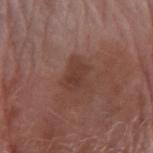Recorded during total-body skin imaging; not selected for excision or biopsy. From the arm. Captured under white-light illumination. Longest diameter approximately 3.5 mm. A female subject, roughly 70 years of age. A region of skin cropped from a whole-body photographic capture, roughly 15 mm wide. The total-body-photography lesion software estimated a footprint of about 7 mm². The analysis additionally found a nevus-likeness score of about 0/100 and a lesion-detection confidence of about 100/100.